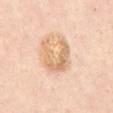{
  "biopsy_status": "not biopsied; imaged during a skin examination",
  "patient": {
    "sex": "male",
    "age_approx": 55
  },
  "lesion_size": {
    "long_diameter_mm_approx": 4.0
  },
  "automated_metrics": {
    "area_mm2_approx": 11.0,
    "shape_asymmetry": 0.5,
    "border_irregularity_0_10": 7.0,
    "color_variation_0_10": 5.0
  },
  "image": {
    "source": "total-body photography crop",
    "field_of_view_mm": 15
  },
  "lighting": "cross-polarized",
  "site": "abdomen"
}